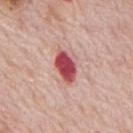{"biopsy_status": "not biopsied; imaged during a skin examination", "automated_metrics": {"cielab_L": 52, "cielab_a": 34, "cielab_b": 23, "vs_skin_contrast_norm": 12.0}, "patient": {"sex": "male", "age_approx": 75}, "site": "mid back", "image": {"source": "total-body photography crop", "field_of_view_mm": 15}, "lighting": "white-light"}